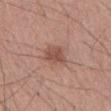This lesion was catalogued during total-body skin photography and was not selected for biopsy. From the abdomen. A male subject aged around 55. An algorithmic analysis of the crop reported an average lesion color of about L≈50 a*≈23 b*≈26 (CIELAB) and a lesion-to-skin contrast of about 7 (normalized; higher = more distinct). The analysis additionally found a detector confidence of about 100 out of 100 that the crop contains a lesion. Cropped from a whole-body photographic skin survey; the tile spans about 15 mm. Measured at roughly 3 mm in maximum diameter.